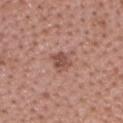biopsy_status: not biopsied; imaged during a skin examination
lighting: white-light
automated_metrics:
  border_irregularity_0_10: 2.5
  color_variation_0_10: 3.0
  peripheral_color_asymmetry: 1.0
  nevus_likeness_0_100: 30
  lesion_detection_confidence_0_100: 100
image:
  source: total-body photography crop
  field_of_view_mm: 15
site: head or neck
lesion_size:
  long_diameter_mm_approx: 2.5
patient:
  sex: male
  age_approx: 40